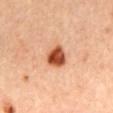The lesion was tiled from a total-body skin photograph and was not biopsied. About 3 mm across. This is a cross-polarized tile. Cropped from a total-body skin-imaging series; the visible field is about 15 mm. Automated image analysis of the tile measured an average lesion color of about L≈52 a*≈30 b*≈37 (CIELAB), roughly 19 lightness units darker than nearby skin, and a normalized lesion–skin contrast near 12.5. It also reported a nevus-likeness score of about 100/100 and lesion-presence confidence of about 100/100. The lesion is located on the mid back. The subject is a female aged 43–47.